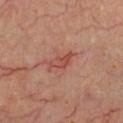  biopsy_status: not biopsied; imaged during a skin examination
  automated_metrics:
    eccentricity: 0.85
    cielab_L: 46
    cielab_a: 27
    cielab_b: 26
    vs_skin_darker_L: 8.0
    vs_skin_contrast_norm: 6.0
  lesion_size:
    long_diameter_mm_approx: 3.0
  image:
    source: total-body photography crop
    field_of_view_mm: 15
  patient:
    sex: male
    age_approx: 60
  lighting: cross-polarized
  site: chest